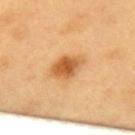Findings:
• notes: no biopsy performed (imaged during a skin exam)
• lesion size: about 4 mm
• TBP lesion metrics: a footprint of about 8 mm², an eccentricity of roughly 0.8, and two-axis asymmetry of about 0.2; a mean CIELAB color near L≈61 a*≈25 b*≈46, roughly 14 lightness units darker than nearby skin, and a lesion-to-skin contrast of about 9 (normalized; higher = more distinct); a border-irregularity index near 2/10, a within-lesion color-variation index near 4/10, and a peripheral color-asymmetry measure near 1; a classifier nevus-likeness of about 95/100 and lesion-presence confidence of about 100/100
• subject: female, roughly 60 years of age
• location: the upper back
• illumination: cross-polarized
• acquisition: ~15 mm crop, total-body skin-cancer survey